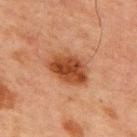Clinical impression: Part of a total-body skin-imaging series; this lesion was reviewed on a skin check and was not flagged for biopsy. Background: A male subject, in their mid- to late 60s. The lesion is located on the chest. Automated tile analysis of the lesion measured a nevus-likeness score of about 95/100 and lesion-presence confidence of about 100/100. Approximately 5 mm at its widest. A close-up tile cropped from a whole-body skin photograph, about 15 mm across. This is a cross-polarized tile.